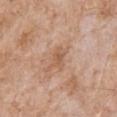No biopsy was performed on this lesion — it was imaged during a full skin examination and was not determined to be concerning. The recorded lesion diameter is about 2.5 mm. A 15 mm crop from a total-body photograph taken for skin-cancer surveillance. A male patient, approximately 65 years of age. Located on the chest. This is a white-light tile.